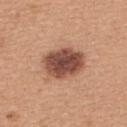The lesion was photographed on a routine skin check and not biopsied; there is no pathology result.
Approximately 5 mm at its widest.
A female patient aged 28–32.
Automated tile analysis of the lesion measured an area of roughly 15 mm², an eccentricity of roughly 0.7, and a symmetry-axis asymmetry near 0.15.
From the back.
This is a white-light tile.
A 15 mm crop from a total-body photograph taken for skin-cancer surveillance.